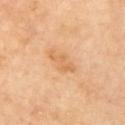Recorded during total-body skin imaging; not selected for excision or biopsy. A female patient in their mid-60s. On the left upper arm. The lesion's longest dimension is about 3.5 mm. The tile uses cross-polarized illumination. Cropped from a whole-body photographic skin survey; the tile spans about 15 mm.